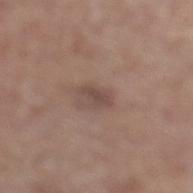Impression:
No biopsy was performed on this lesion — it was imaged during a full skin examination and was not determined to be concerning.
Image and clinical context:
A region of skin cropped from a whole-body photographic capture, roughly 15 mm wide. Captured under white-light illumination. The lesion is on the left lower leg. Measured at roughly 3 mm in maximum diameter. The subject is a male aged approximately 65.